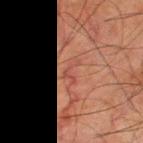Part of a total-body skin-imaging series; this lesion was reviewed on a skin check and was not flagged for biopsy. Imaged with cross-polarized lighting. This image is a 15 mm lesion crop taken from a total-body photograph. On the left thigh. A male subject, aged 78 to 82. About 3 mm across. Automated image analysis of the tile measured an eccentricity of roughly 0.85 and a shape-asymmetry score of about 0.65 (0 = symmetric). And it measured an average lesion color of about L≈39 a*≈22 b*≈24 (CIELAB) and a normalized lesion–skin contrast near 4.5. It also reported border irregularity of about 7 on a 0–10 scale, internal color variation of about 0 on a 0–10 scale, and a peripheral color-asymmetry measure near 0. And it measured a classifier nevus-likeness of about 0/100 and a detector confidence of about 60 out of 100 that the crop contains a lesion.